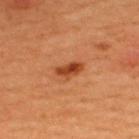follow-up: total-body-photography surveillance lesion; no biopsy | lesion size: ~3.5 mm (longest diameter) | subject: male, in their mid- to late 60s | lighting: cross-polarized | body site: the back | image source: total-body-photography crop, ~15 mm field of view.